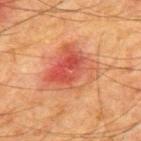Clinical impression:
This lesion was catalogued during total-body skin photography and was not selected for biopsy.
Background:
The lesion is located on the upper back. The patient is a male aged 63 to 67. The tile uses cross-polarized illumination. A 15 mm crop from a total-body photograph taken for skin-cancer surveillance. Automated image analysis of the tile measured an area of roughly 23 mm², an eccentricity of roughly 0.6, and two-axis asymmetry of about 0.3. The software also gave internal color variation of about 9.5 on a 0–10 scale. And it measured an automated nevus-likeness rating near 5 out of 100 and lesion-presence confidence of about 100/100.